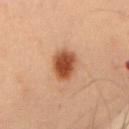A male subject about 55 years old.
The lesion is on the back.
A lesion tile, about 15 mm wide, cut from a 3D total-body photograph.